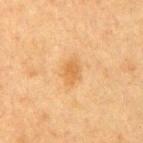Assessment: Imaged during a routine full-body skin examination; the lesion was not biopsied and no histopathology is available. Acquisition and patient details: This image is a 15 mm lesion crop taken from a total-body photograph. A female patient, approximately 40 years of age. Approximately 3 mm at its widest. The lesion is on the right upper arm. Captured under cross-polarized illumination.